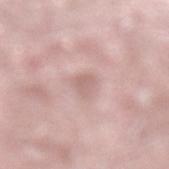Clinical impression: Captured during whole-body skin photography for melanoma surveillance; the lesion was not biopsied. Clinical summary: The lesion is on the left lower leg. A male subject aged approximately 75. A lesion tile, about 15 mm wide, cut from a 3D total-body photograph.